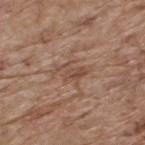Imaged during a routine full-body skin examination; the lesion was not biopsied and no histopathology is available.
A male patient, roughly 70 years of age.
The lesion is on the back.
Cropped from a whole-body photographic skin survey; the tile spans about 15 mm.